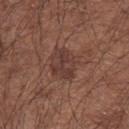Q: Was a biopsy performed?
A: no biopsy performed (imaged during a skin exam)
Q: Who is the patient?
A: male, about 65 years old
Q: Lesion location?
A: the left upper arm
Q: How was the tile lit?
A: white-light
Q: What did automated image analysis measure?
A: border irregularity of about 3 on a 0–10 scale and radial color variation of about 1.5
Q: What is the lesion's diameter?
A: about 4 mm
Q: How was this image acquired?
A: ~15 mm crop, total-body skin-cancer survey